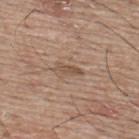A lesion tile, about 15 mm wide, cut from a 3D total-body photograph. Imaged with white-light lighting. From the back. The lesion's longest dimension is about 3 mm. A male patient, aged approximately 65. The lesion-visualizer software estimated two-axis asymmetry of about 0.3. And it measured an average lesion color of about L≈51 a*≈16 b*≈28 (CIELAB), a lesion–skin lightness drop of about 9, and a lesion-to-skin contrast of about 6.5 (normalized; higher = more distinct). The software also gave a classifier nevus-likeness of about 0/100 and a detector confidence of about 100 out of 100 that the crop contains a lesion.